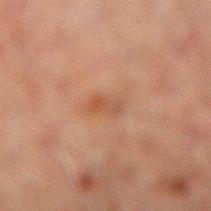notes = imaged on a skin check; not biopsied